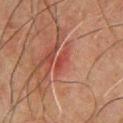| field | value |
|---|---|
| follow-up | no biopsy performed (imaged during a skin exam) |
| acquisition | 15 mm crop, total-body photography |
| body site | the front of the torso |
| automated metrics | an average lesion color of about L≈37 a*≈28 b*≈25 (CIELAB) and about 8 CIELAB-L* units darker than the surrounding skin; a peripheral color-asymmetry measure near 0; a lesion-detection confidence of about 65/100 |
| subject | male, roughly 65 years of age |